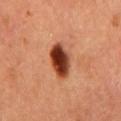The lesion was tiled from a total-body skin photograph and was not biopsied. Captured under cross-polarized illumination. The lesion's longest dimension is about 5 mm. From the chest. A male patient approximately 65 years of age. Cropped from a total-body skin-imaging series; the visible field is about 15 mm.